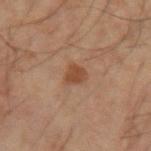Part of a total-body skin-imaging series; this lesion was reviewed on a skin check and was not flagged for biopsy. From the right forearm. A 15 mm crop from a total-body photograph taken for skin-cancer surveillance. The patient is a male roughly 60 years of age.